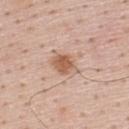| key | value |
|---|---|
| workup | no biopsy performed (imaged during a skin exam) |
| automated metrics | a mean CIELAB color near L≈60 a*≈20 b*≈31, roughly 11 lightness units darker than nearby skin, and a lesion-to-skin contrast of about 8 (normalized; higher = more distinct); a within-lesion color-variation index near 3/10 and radial color variation of about 1; an automated nevus-likeness rating near 95 out of 100 and a lesion-detection confidence of about 100/100 |
| tile lighting | white-light illumination |
| lesion diameter | about 3.5 mm |
| anatomic site | the back |
| patient | male, aged 58 to 62 |
| acquisition | ~15 mm tile from a whole-body skin photo |